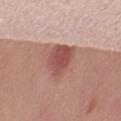• notes — catalogued during a skin exam; not biopsied
• image-analysis metrics — an average lesion color of about L≈51 a*≈26 b*≈24 (CIELAB), roughly 12 lightness units darker than nearby skin, and a normalized border contrast of about 8
• illumination — white-light illumination
• subject — female, about 50 years old
• image source — 15 mm crop, total-body photography
• anatomic site — the right thigh
• lesion size — ~4.5 mm (longest diameter)